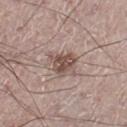* follow-up: no biopsy performed (imaged during a skin exam)
* location: the left leg
* image source: ~15 mm crop, total-body skin-cancer survey
* lighting: white-light illumination
* size: ≈3.5 mm
* subject: male, in their 70s